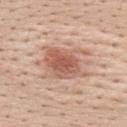* workup — imaged on a skin check; not biopsied
* image-analysis metrics — a lesion color around L≈59 a*≈22 b*≈29 in CIELAB, a lesion–skin lightness drop of about 12, and a lesion-to-skin contrast of about 7.5 (normalized; higher = more distinct); a lesion-detection confidence of about 100/100
* lesion size — about 5.5 mm
* acquisition — ~15 mm tile from a whole-body skin photo
* anatomic site — the chest
* lighting — white-light
* patient — female, in their mid-20s